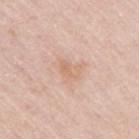Captured during whole-body skin photography for melanoma surveillance; the lesion was not biopsied.
The lesion is on the right upper arm.
Captured under white-light illumination.
A 15 mm close-up tile from a total-body photography series done for melanoma screening.
Automated tile analysis of the lesion measured a mean CIELAB color near L≈67 a*≈19 b*≈32 and a normalized lesion–skin contrast near 5. And it measured a border-irregularity rating of about 3.5/10, a within-lesion color-variation index near 3/10, and peripheral color asymmetry of about 1.
A female patient about 65 years old.